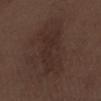<lesion>
<biopsy_status>not biopsied; imaged during a skin examination</biopsy_status>
<automated_metrics>
  <cielab_L>27</cielab_L>
  <cielab_a>15</cielab_a>
  <cielab_b>19</cielab_b>
  <vs_skin_darker_L>5.0</vs_skin_darker_L>
  <border_irregularity_0_10>5.0</border_irregularity_0_10>
</automated_metrics>
<image>
  <source>total-body photography crop</source>
  <field_of_view_mm>15</field_of_view_mm>
</image>
<patient>
  <sex>male</sex>
  <age_approx>70</age_approx>
</patient>
<lesion_size>
  <long_diameter_mm_approx>7.5</long_diameter_mm_approx>
</lesion_size>
<lighting>white-light</lighting>
<site>arm</site>
</lesion>